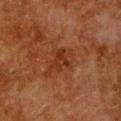Imaged during a routine full-body skin examination; the lesion was not biopsied and no histopathology is available. The subject is a female aged 48–52. A 15 mm close-up extracted from a 3D total-body photography capture. On the chest.